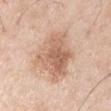Recorded during total-body skin imaging; not selected for excision or biopsy. The tile uses white-light illumination. Cropped from a total-body skin-imaging series; the visible field is about 15 mm. The subject is a male aged 63 to 67. The lesion is on the right upper arm.